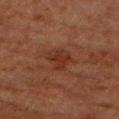Imaged during a routine full-body skin examination; the lesion was not biopsied and no histopathology is available. A close-up tile cropped from a whole-body skin photograph, about 15 mm across. A male patient roughly 80 years of age. The tile uses cross-polarized illumination. Located on the right upper arm. The lesion-visualizer software estimated a lesion–skin lightness drop of about 5 and a lesion-to-skin contrast of about 6 (normalized; higher = more distinct). And it measured a border-irregularity index near 3/10, a color-variation rating of about 2/10, and peripheral color asymmetry of about 0.5.